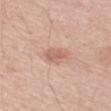Clinical impression:
Imaged during a routine full-body skin examination; the lesion was not biopsied and no histopathology is available.
Clinical summary:
About 3 mm across. Captured under white-light illumination. On the right upper arm. A region of skin cropped from a whole-body photographic capture, roughly 15 mm wide. The patient is a male aged approximately 40. An algorithmic analysis of the crop reported border irregularity of about 3.5 on a 0–10 scale and a within-lesion color-variation index near 3/10. And it measured a nevus-likeness score of about 5/100 and lesion-presence confidence of about 100/100.